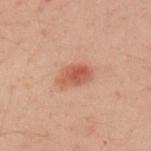Captured during whole-body skin photography for melanoma surveillance; the lesion was not biopsied.
A close-up tile cropped from a whole-body skin photograph, about 15 mm across.
An algorithmic analysis of the crop reported an area of roughly 7 mm² and a shape eccentricity near 0.8. The software also gave a border-irregularity rating of about 2/10, a color-variation rating of about 3.5/10, and radial color variation of about 1.
Located on the right upper arm.
A male subject roughly 40 years of age.
Captured under cross-polarized illumination.